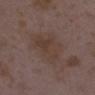follow-up: imaged on a skin check; not biopsied
patient: female, in their mid- to late 30s
illumination: white-light illumination
imaging modality: ~15 mm crop, total-body skin-cancer survey
site: the right lower leg